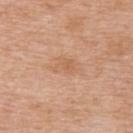lesion size = ≈4 mm
imaging modality = 15 mm crop, total-body photography
body site = the upper back
subject = female, about 65 years old